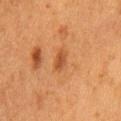follow-up = no biopsy performed (imaged during a skin exam); lesion size = ≈2.5 mm; automated metrics = a border-irregularity index near 2/10 and peripheral color asymmetry of about 0.5; anatomic site = the chest; lighting = cross-polarized illumination; subject = female, in their 50s; acquisition = ~15 mm crop, total-body skin-cancer survey.